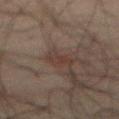{
  "biopsy_status": "not biopsied; imaged during a skin examination",
  "patient": {
    "sex": "male",
    "age_approx": 70
  },
  "site": "front of the torso",
  "image": {
    "source": "total-body photography crop",
    "field_of_view_mm": 15
  },
  "lesion_size": {
    "long_diameter_mm_approx": 3.0
  },
  "automated_metrics": {
    "area_mm2_approx": 5.5,
    "eccentricity": 0.4,
    "shape_asymmetry": 0.4,
    "cielab_L": 29,
    "cielab_a": 13,
    "cielab_b": 18,
    "vs_skin_darker_L": 5.0,
    "vs_skin_contrast_norm": 6.0,
    "border_irregularity_0_10": 4.0,
    "color_variation_0_10": 2.0,
    "peripheral_color_asymmetry": 0.5,
    "nevus_likeness_0_100": 50,
    "lesion_detection_confidence_0_100": 100
  }
}